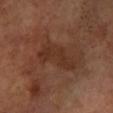This lesion was catalogued during total-body skin photography and was not selected for biopsy. From the left lower leg. A male subject approximately 65 years of age. Automated tile analysis of the lesion measured a within-lesion color-variation index near 2.5/10 and radial color variation of about 1. Captured under cross-polarized illumination. The lesion's longest dimension is about 6 mm. A close-up tile cropped from a whole-body skin photograph, about 15 mm across.